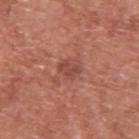The lesion was photographed on a routine skin check and not biopsied; there is no pathology result. On the back. Imaged with white-light lighting. A roughly 15 mm field-of-view crop from a total-body skin photograph. Longest diameter approximately 2.5 mm. A male patient about 75 years old.